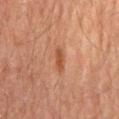| key | value |
|---|---|
| workup | total-body-photography surveillance lesion; no biopsy |
| patient | male, aged 58–62 |
| image | ~15 mm crop, total-body skin-cancer survey |
| site | the mid back |
| illumination | cross-polarized illumination |
| diameter | ≈3 mm |